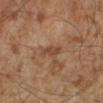Assessment: The lesion was tiled from a total-body skin photograph and was not biopsied. Image and clinical context: A male patient aged 58–62. On the right lower leg. A 15 mm close-up tile from a total-body photography series done for melanoma screening.